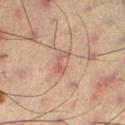Part of a total-body skin-imaging series; this lesion was reviewed on a skin check and was not flagged for biopsy. The tile uses cross-polarized illumination. A male patient, about 60 years old. Approximately 3 mm at its widest. The lesion-visualizer software estimated an average lesion color of about L≈51 a*≈18 b*≈26 (CIELAB), a lesion–skin lightness drop of about 7, and a normalized lesion–skin contrast near 5. The software also gave internal color variation of about 5 on a 0–10 scale and peripheral color asymmetry of about 2. A 15 mm crop from a total-body photograph taken for skin-cancer surveillance.